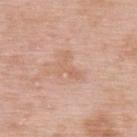This lesion was catalogued during total-body skin photography and was not selected for biopsy. The recorded lesion diameter is about 3.5 mm. A 15 mm crop from a total-body photograph taken for skin-cancer surveillance. The lesion is located on the upper back. The subject is a female aged around 50. The tile uses white-light illumination.